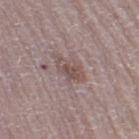Recorded during total-body skin imaging; not selected for excision or biopsy.
Captured under white-light illumination.
The patient is a female about 55 years old.
This image is a 15 mm lesion crop taken from a total-body photograph.
The lesion is located on the right thigh.
An algorithmic analysis of the crop reported a lesion area of about 7 mm², an eccentricity of roughly 0.8, and a symmetry-axis asymmetry near 0.3. And it measured a nevus-likeness score of about 5/100 and a lesion-detection confidence of about 75/100.
About 4.5 mm across.